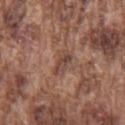Impression:
Captured during whole-body skin photography for melanoma surveillance; the lesion was not biopsied.
Image and clinical context:
Imaged with white-light lighting. On the chest. A region of skin cropped from a whole-body photographic capture, roughly 15 mm wide. A male subject, aged around 75. The recorded lesion diameter is about 3 mm.